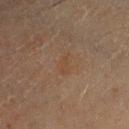Clinical impression: Recorded during total-body skin imaging; not selected for excision or biopsy. Acquisition and patient details: Imaged with cross-polarized lighting. This image is a 15 mm lesion crop taken from a total-body photograph. A female subject, about 40 years old. The recorded lesion diameter is about 3 mm. The lesion is located on the head or neck.